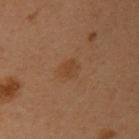Notes:
* follow-up — no biopsy performed (imaged during a skin exam)
* tile lighting — cross-polarized
* anatomic site — the chest
* automated lesion analysis — a normalized border contrast of about 5; a detector confidence of about 100 out of 100 that the crop contains a lesion
* imaging modality — ~15 mm crop, total-body skin-cancer survey
* patient — female, about 50 years old